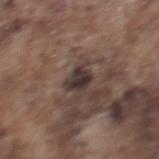Assessment:
This lesion was catalogued during total-body skin photography and was not selected for biopsy.
Clinical summary:
The tile uses white-light illumination. On the mid back. A male subject aged around 75. A roughly 15 mm field-of-view crop from a total-body skin photograph.